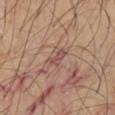Captured under cross-polarized illumination.
Approximately 2.5 mm at its widest.
From the chest.
A subject aged approximately 65.
The lesion-visualizer software estimated an area of roughly 3 mm², an eccentricity of roughly 0.85, and a shape-asymmetry score of about 0.3 (0 = symmetric).
A lesion tile, about 15 mm wide, cut from a 3D total-body photograph.